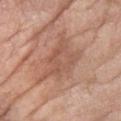Assessment:
Captured during whole-body skin photography for melanoma surveillance; the lesion was not biopsied.
Context:
A 15 mm close-up extracted from a 3D total-body photography capture. The patient is a female in their mid-60s. Captured under white-light illumination. About 7.5 mm across. Located on the left forearm.